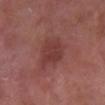Impression:
This lesion was catalogued during total-body skin photography and was not selected for biopsy.
Clinical summary:
A male subject roughly 65 years of age. On the right lower leg. A 15 mm crop from a total-body photograph taken for skin-cancer surveillance. Captured under white-light illumination. Approximately 4 mm at its widest. An algorithmic analysis of the crop reported an area of roughly 10 mm² and a shape eccentricity near 0.65. The analysis additionally found a border-irregularity rating of about 3/10, a within-lesion color-variation index near 2/10, and peripheral color asymmetry of about 1.